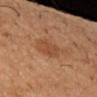The subject is a male aged approximately 55.
This is a cross-polarized tile.
A region of skin cropped from a whole-body photographic capture, roughly 15 mm wide.
Measured at roughly 3 mm in maximum diameter.
The lesion is located on the left upper arm.
The lesion-visualizer software estimated an outline eccentricity of about 0.55 (0 = round, 1 = elongated) and a symmetry-axis asymmetry near 0.25. The analysis additionally found border irregularity of about 2.5 on a 0–10 scale and a peripheral color-asymmetry measure near 0.5. It also reported a nevus-likeness score of about 25/100 and a lesion-detection confidence of about 100/100.